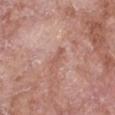| field | value |
|---|---|
| notes | no biopsy performed (imaged during a skin exam) |
| body site | the chest |
| image-analysis metrics | a classifier nevus-likeness of about 0/100 and lesion-presence confidence of about 100/100 |
| tile lighting | white-light illumination |
| imaging modality | ~15 mm crop, total-body skin-cancer survey |
| patient | female, aged approximately 75 |